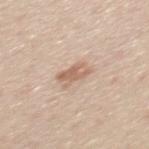biopsy status = catalogued during a skin exam; not biopsied
image source = ~15 mm crop, total-body skin-cancer survey
patient = male, in their mid- to late 30s
site = the back
lesion diameter = ≈3 mm